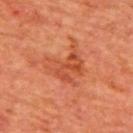Imaged during a routine full-body skin examination; the lesion was not biopsied and no histopathology is available. The lesion is located on the back. A lesion tile, about 15 mm wide, cut from a 3D total-body photograph. Captured under cross-polarized illumination. A male subject approximately 65 years of age. Longest diameter approximately 5 mm.